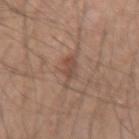Impression:
No biopsy was performed on this lesion — it was imaged during a full skin examination and was not determined to be concerning.
Context:
On the right forearm. The patient is a male aged around 35. Automated image analysis of the tile measured roughly 8 lightness units darker than nearby skin and a lesion-to-skin contrast of about 6 (normalized; higher = more distinct). A 15 mm close-up extracted from a 3D total-body photography capture. Longest diameter approximately 3.5 mm.